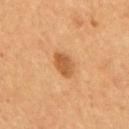Impression: The lesion was tiled from a total-body skin photograph and was not biopsied. Background: On the mid back. A male subject aged approximately 55. A 15 mm close-up extracted from a 3D total-body photography capture. Approximately 3.5 mm at its widest.